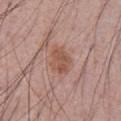Q: Was this lesion biopsied?
A: catalogued during a skin exam; not biopsied
Q: Illumination type?
A: white-light illumination
Q: Where on the body is the lesion?
A: the abdomen
Q: How large is the lesion?
A: ~4 mm (longest diameter)
Q: What kind of image is this?
A: 15 mm crop, total-body photography
Q: What are the patient's age and sex?
A: male, roughly 70 years of age